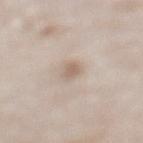This lesion was catalogued during total-body skin photography and was not selected for biopsy. A male patient about 80 years old. About 3 mm across. This is a white-light tile. Located on the left lower leg. A close-up tile cropped from a whole-body skin photograph, about 15 mm across.